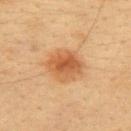The lesion was tiled from a total-body skin photograph and was not biopsied. A 15 mm close-up tile from a total-body photography series done for melanoma screening. This is a cross-polarized tile. Measured at roughly 4.5 mm in maximum diameter. The lesion is on the upper back. A female subject in their 30s.